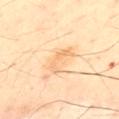The lesion was tiled from a total-body skin photograph and was not biopsied.
Longest diameter approximately 4 mm.
The lesion is located on the upper back.
This is a cross-polarized tile.
The total-body-photography lesion software estimated an average lesion color of about L≈76 a*≈20 b*≈42 (CIELAB), about 8 CIELAB-L* units darker than the surrounding skin, and a lesion-to-skin contrast of about 5 (normalized; higher = more distinct). The analysis additionally found an automated nevus-likeness rating near 0 out of 100 and lesion-presence confidence of about 100/100.
A male patient approximately 50 years of age.
A region of skin cropped from a whole-body photographic capture, roughly 15 mm wide.